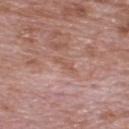This lesion was catalogued during total-body skin photography and was not selected for biopsy. A region of skin cropped from a whole-body photographic capture, roughly 15 mm wide. Located on the upper back. The patient is a male about 70 years old.